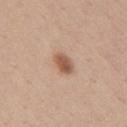notes — imaged on a skin check; not biopsied | lesion diameter — ~3 mm (longest diameter) | image — ~15 mm crop, total-body skin-cancer survey | site — the mid back | subject — female, about 40 years old | lighting — white-light illumination.